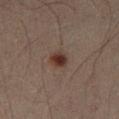Q: Was a biopsy performed?
A: imaged on a skin check; not biopsied
Q: What are the patient's age and sex?
A: male, aged 38–42
Q: What kind of image is this?
A: 15 mm crop, total-body photography
Q: What is the anatomic site?
A: the left thigh